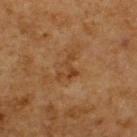No biopsy was performed on this lesion — it was imaged during a full skin examination and was not determined to be concerning. The lesion is located on the upper back. Cropped from a whole-body photographic skin survey; the tile spans about 15 mm. The subject is a male aged approximately 60.